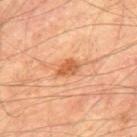Impression:
Captured during whole-body skin photography for melanoma surveillance; the lesion was not biopsied.
Context:
Cropped from a total-body skin-imaging series; the visible field is about 15 mm. Longest diameter approximately 3 mm. A male patient in their mid-60s. The lesion is located on the mid back. Imaged with cross-polarized lighting. The lesion-visualizer software estimated a border-irregularity rating of about 2/10 and peripheral color asymmetry of about 1. The software also gave a classifier nevus-likeness of about 85/100 and lesion-presence confidence of about 100/100.